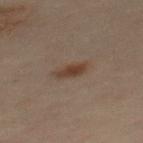Impression:
Part of a total-body skin-imaging series; this lesion was reviewed on a skin check and was not flagged for biopsy.
Acquisition and patient details:
A region of skin cropped from a whole-body photographic capture, roughly 15 mm wide. Located on the back. The patient is a male aged 48–52.